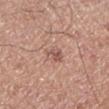Impression: Part of a total-body skin-imaging series; this lesion was reviewed on a skin check and was not flagged for biopsy. Background: The lesion-visualizer software estimated a lesion color around L≈55 a*≈20 b*≈26 in CIELAB, about 8 CIELAB-L* units darker than the surrounding skin, and a lesion-to-skin contrast of about 6 (normalized; higher = more distinct). And it measured a border-irregularity rating of about 2.5/10, a within-lesion color-variation index near 4.5/10, and a peripheral color-asymmetry measure near 1.5. And it measured an automated nevus-likeness rating near 0 out of 100 and lesion-presence confidence of about 100/100. A male subject approximately 50 years of age. This is a white-light tile. Cropped from a total-body skin-imaging series; the visible field is about 15 mm. On the right lower leg. Measured at roughly 3 mm in maximum diameter.